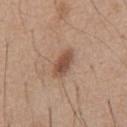Clinical impression: The lesion was tiled from a total-body skin photograph and was not biopsied. Acquisition and patient details: The subject is a male roughly 55 years of age. About 4 mm across. On the chest. A 15 mm close-up extracted from a 3D total-body photography capture.